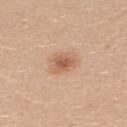The lesion was tiled from a total-body skin photograph and was not biopsied.
From the back.
Automated image analysis of the tile measured an outline eccentricity of about 0.6 (0 = round, 1 = elongated) and two-axis asymmetry of about 0.25. The analysis additionally found a mean CIELAB color near L≈59 a*≈22 b*≈32, roughly 11 lightness units darker than nearby skin, and a lesion-to-skin contrast of about 7 (normalized; higher = more distinct). The analysis additionally found a detector confidence of about 100 out of 100 that the crop contains a lesion.
The recorded lesion diameter is about 3 mm.
The patient is a female roughly 25 years of age.
Cropped from a whole-body photographic skin survey; the tile spans about 15 mm.
This is a white-light tile.